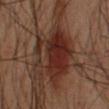Q: How was this image acquired?
A: ~15 mm tile from a whole-body skin photo
Q: Lesion location?
A: the left arm
Q: What are the patient's age and sex?
A: male, about 50 years old